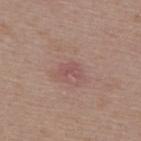This lesion was catalogued during total-body skin photography and was not selected for biopsy. Located on the upper back. The lesion's longest dimension is about 2.5 mm. The total-body-photography lesion software estimated two-axis asymmetry of about 0.45. And it measured a border-irregularity rating of about 4.5/10. And it measured an automated nevus-likeness rating near 0 out of 100. The tile uses white-light illumination. A close-up tile cropped from a whole-body skin photograph, about 15 mm across. A female patient aged around 45.